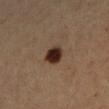| field | value |
|---|---|
| biopsy status | no biopsy performed (imaged during a skin exam) |